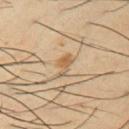diameter: about 3 mm; patient: male, aged 38–42; image source: total-body-photography crop, ~15 mm field of view; location: the left upper arm; illumination: cross-polarized.